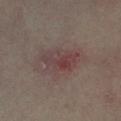| field | value |
|---|---|
| biopsy status | total-body-photography surveillance lesion; no biopsy |
| image | total-body-photography crop, ~15 mm field of view |
| TBP lesion metrics | about 7 CIELAB-L* units darker than the surrounding skin and a normalized border contrast of about 6.5; a border-irregularity index near 3.5/10, a within-lesion color-variation index near 6/10, and radial color variation of about 2; an automated nevus-likeness rating near 5 out of 100 and a lesion-detection confidence of about 100/100 |
| lighting | cross-polarized |
| site | the left lower leg |
| subject | female, approximately 35 years of age |